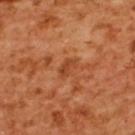Recorded during total-body skin imaging; not selected for excision or biopsy. Approximately 2.5 mm at its widest. A female subject aged 53–57. The tile uses cross-polarized illumination. A roughly 15 mm field-of-view crop from a total-body skin photograph. An algorithmic analysis of the crop reported a footprint of about 3 mm², an outline eccentricity of about 0.85 (0 = round, 1 = elongated), and two-axis asymmetry of about 0.4. It also reported an average lesion color of about L≈47 a*≈30 b*≈41 (CIELAB), about 9 CIELAB-L* units darker than the surrounding skin, and a normalized lesion–skin contrast near 6.5. And it measured an automated nevus-likeness rating near 0 out of 100. Located on the upper back.